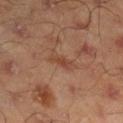{"biopsy_status": "not biopsied; imaged during a skin examination", "image": {"source": "total-body photography crop", "field_of_view_mm": 15}, "lesion_size": {"long_diameter_mm_approx": 2.5}, "patient": {"sex": "male", "age_approx": 45}, "site": "right lower leg"}